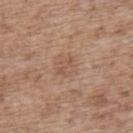Part of a total-body skin-imaging series; this lesion was reviewed on a skin check and was not flagged for biopsy. A male patient, aged 68 to 72. From the upper back. Cropped from a whole-body photographic skin survey; the tile spans about 15 mm. Captured under white-light illumination. About 2.5 mm across.